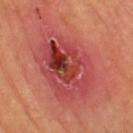Imaged during a routine full-body skin examination; the lesion was not biopsied and no histopathology is available. The lesion is located on the right leg. A female patient, approximately 60 years of age. Cropped from a total-body skin-imaging series; the visible field is about 15 mm.